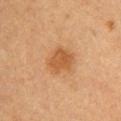Context:
Automated image analysis of the tile measured an area of roughly 8 mm² and a symmetry-axis asymmetry near 0.3. It also reported a nevus-likeness score of about 85/100 and a lesion-detection confidence of about 100/100. Located on the left upper arm. A female subject, aged 38 to 42. The tile uses cross-polarized illumination. The lesion's longest dimension is about 3.5 mm. A region of skin cropped from a whole-body photographic capture, roughly 15 mm wide.